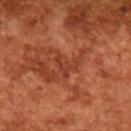notes — catalogued during a skin exam; not biopsied | patient — male, roughly 65 years of age | tile lighting — cross-polarized | lesion diameter — ~3 mm (longest diameter) | imaging modality — total-body-photography crop, ~15 mm field of view.